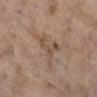Q: Was a biopsy performed?
A: no biopsy performed (imaged during a skin exam)
Q: What are the patient's age and sex?
A: female, roughly 85 years of age or older
Q: What is the imaging modality?
A: total-body-photography crop, ~15 mm field of view
Q: Lesion location?
A: the right lower leg
Q: Lesion size?
A: ~4 mm (longest diameter)
Q: Illumination type?
A: white-light illumination
Q: What did automated image analysis measure?
A: a mean CIELAB color near L≈51 a*≈15 b*≈28, roughly 7 lightness units darker than nearby skin, and a normalized lesion–skin contrast near 5.5; border irregularity of about 8.5 on a 0–10 scale, a within-lesion color-variation index near 3/10, and a peripheral color-asymmetry measure near 1; a nevus-likeness score of about 0/100 and lesion-presence confidence of about 100/100